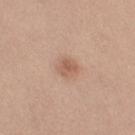| field | value |
|---|---|
| follow-up | catalogued during a skin exam; not biopsied |
| diameter | ≈2.5 mm |
| patient | female, aged 28–32 |
| acquisition | ~15 mm crop, total-body skin-cancer survey |
| location | the left thigh |
| tile lighting | white-light illumination |